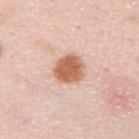Findings:
– follow-up — catalogued during a skin exam; not biopsied
– subject — male, in their mid-30s
– location — the upper back
– imaging modality — ~15 mm crop, total-body skin-cancer survey